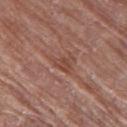Findings:
- biopsy status · no biopsy performed (imaged during a skin exam)
- body site · the left thigh
- size · about 2.5 mm
- automated metrics · a footprint of about 4 mm², an eccentricity of roughly 0.7, and a shape-asymmetry score of about 0.5 (0 = symmetric); a lesion-to-skin contrast of about 6 (normalized; higher = more distinct)
- patient · female, aged 78–82
- image · total-body-photography crop, ~15 mm field of view